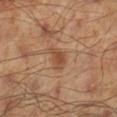Captured during whole-body skin photography for melanoma surveillance; the lesion was not biopsied.